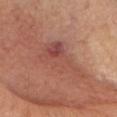– workup — total-body-photography surveillance lesion; no biopsy
– image — ~15 mm crop, total-body skin-cancer survey
– anatomic site — the head or neck
– subject — male, roughly 70 years of age